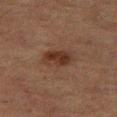| key | value |
|---|---|
| workup | catalogued during a skin exam; not biopsied |
| automated lesion analysis | a lesion color around L≈27 a*≈17 b*≈23 in CIELAB, a lesion–skin lightness drop of about 8, and a normalized border contrast of about 8.5; internal color variation of about 3.5 on a 0–10 scale and a peripheral color-asymmetry measure near 1 |
| patient | female, aged 58–62 |
| image source | ~15 mm tile from a whole-body skin photo |
| illumination | cross-polarized illumination |
| site | the right thigh |
| size | ~3.5 mm (longest diameter) |